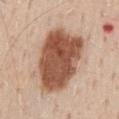– biopsy status: total-body-photography surveillance lesion; no biopsy
– site: the mid back
– patient: male, roughly 60 years of age
– image source: ~15 mm crop, total-body skin-cancer survey
– image-analysis metrics: an average lesion color of about L≈52 a*≈22 b*≈31 (CIELAB), a lesion–skin lightness drop of about 19, and a normalized border contrast of about 12.5; a within-lesion color-variation index near 5/10 and a peripheral color-asymmetry measure near 1.5; a classifier nevus-likeness of about 95/100
– diameter: about 8 mm